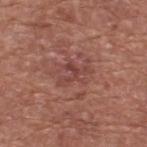Clinical impression: No biopsy was performed on this lesion — it was imaged during a full skin examination and was not determined to be concerning. Image and clinical context: Located on the upper back. A lesion tile, about 15 mm wide, cut from a 3D total-body photograph. Imaged with white-light lighting. A male subject, approximately 75 years of age. The lesion-visualizer software estimated a footprint of about 5.5 mm², an eccentricity of roughly 0.75, and two-axis asymmetry of about 0.65. It also reported a mean CIELAB color near L≈44 a*≈25 b*≈24. The analysis additionally found a nevus-likeness score of about 0/100 and lesion-presence confidence of about 90/100. Measured at roughly 4 mm in maximum diameter.